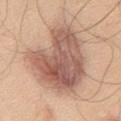Impression:
This lesion was catalogued during total-body skin photography and was not selected for biopsy.
Image and clinical context:
About 9.5 mm across. The lesion is located on the chest. The total-body-photography lesion software estimated an area of roughly 43 mm², a shape eccentricity near 0.7, and a shape-asymmetry score of about 0.35 (0 = symmetric). The analysis additionally found a lesion–skin lightness drop of about 14 and a lesion-to-skin contrast of about 9.5 (normalized; higher = more distinct). And it measured a color-variation rating of about 6.5/10 and a peripheral color-asymmetry measure near 2. A male patient, aged 43–47. A region of skin cropped from a whole-body photographic capture, roughly 15 mm wide.